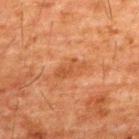Impression: The lesion was tiled from a total-body skin photograph and was not biopsied. Context: Automated image analysis of the tile measured an average lesion color of about L≈41 a*≈23 b*≈33 (CIELAB), a lesion–skin lightness drop of about 6, and a normalized border contrast of about 5.5. And it measured a border-irregularity index near 5/10, a color-variation rating of about 1.5/10, and peripheral color asymmetry of about 0.5. The software also gave a nevus-likeness score of about 0/100 and a lesion-detection confidence of about 100/100. Captured under cross-polarized illumination. The subject is a male aged approximately 60. On the back. Measured at roughly 4 mm in maximum diameter. A 15 mm close-up tile from a total-body photography series done for melanoma screening.